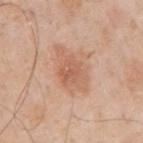{"biopsy_status": "not biopsied; imaged during a skin examination", "image": {"source": "total-body photography crop", "field_of_view_mm": 15}, "site": "right upper arm", "patient": {"sex": "male", "age_approx": 70}, "lesion_size": {"long_diameter_mm_approx": 6.0}, "lighting": "white-light"}